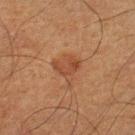Q: Was this lesion biopsied?
A: catalogued during a skin exam; not biopsied
Q: What lighting was used for the tile?
A: cross-polarized
Q: What kind of image is this?
A: ~15 mm tile from a whole-body skin photo
Q: Automated lesion metrics?
A: an area of roughly 7 mm², an outline eccentricity of about 0.4 (0 = round, 1 = elongated), and two-axis asymmetry of about 0.4; an automated nevus-likeness rating near 10 out of 100 and a detector confidence of about 100 out of 100 that the crop contains a lesion
Q: Patient demographics?
A: male, aged approximately 65
Q: Lesion location?
A: the right lower leg
Q: What is the lesion's diameter?
A: ≈3.5 mm